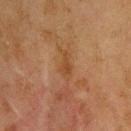Case summary:
• acquisition · ~15 mm crop, total-body skin-cancer survey
• diameter · ~3 mm (longest diameter)
• patient · male, aged 43 to 47
• site · the upper back